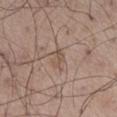biopsy_status: not biopsied; imaged during a skin examination
patient:
  sex: male
  age_approx: 55
site: right thigh
image:
  source: total-body photography crop
  field_of_view_mm: 15